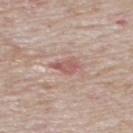follow-up: total-body-photography surveillance lesion; no biopsy
subject: male, aged around 75
acquisition: ~15 mm crop, total-body skin-cancer survey
size: ≈2.5 mm
anatomic site: the mid back